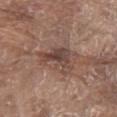Q: Was a biopsy performed?
A: total-body-photography surveillance lesion; no biopsy
Q: What is the imaging modality?
A: total-body-photography crop, ~15 mm field of view
Q: What are the patient's age and sex?
A: male, aged approximately 80
Q: Where on the body is the lesion?
A: the abdomen